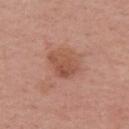Findings:
* notes — catalogued during a skin exam; not biopsied
* image — 15 mm crop, total-body photography
* lesion diameter — ≈4 mm
* lighting — white-light illumination
* patient — female, roughly 55 years of age
* anatomic site — the left upper arm